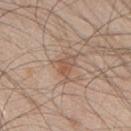Context:
An algorithmic analysis of the crop reported an average lesion color of about L≈55 a*≈17 b*≈28 (CIELAB) and a lesion–skin lightness drop of about 8. The analysis additionally found a nevus-likeness score of about 0/100 and a detector confidence of about 100 out of 100 that the crop contains a lesion. A male subject, aged approximately 50. The lesion is on the upper back. Captured under white-light illumination. Cropped from a whole-body photographic skin survey; the tile spans about 15 mm. About 3.5 mm across.